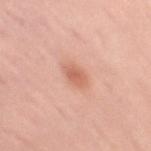biopsy status: no biopsy performed (imaged during a skin exam) | body site: the right upper arm | tile lighting: white-light illumination | imaging modality: 15 mm crop, total-body photography | subject: female, aged 48 to 52.